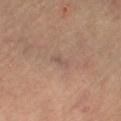- workup · catalogued during a skin exam; not biopsied
- TBP lesion metrics · a lesion area of about 3 mm² and two-axis asymmetry of about 0.3; a border-irregularity index near 3/10, internal color variation of about 0.5 on a 0–10 scale, and peripheral color asymmetry of about 0; a detector confidence of about 65 out of 100 that the crop contains a lesion
- location · the leg
- image · ~15 mm crop, total-body skin-cancer survey
- patient · female, roughly 65 years of age